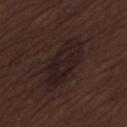Case summary:
- image · 15 mm crop, total-body photography
- illumination · white-light
- body site · the lower back
- TBP lesion metrics · a lesion color around L≈18 a*≈14 b*≈14 in CIELAB, roughly 5 lightness units darker than nearby skin, and a normalized border contrast of about 8.5
- diameter · about 6.5 mm
- subject · male, in their 70s